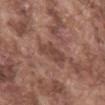biopsy status: no biopsy performed (imaged during a skin exam); lesion diameter: ~4 mm (longest diameter); illumination: white-light; image: total-body-photography crop, ~15 mm field of view; subject: male, in their mid- to late 70s; site: the mid back.